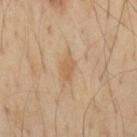Imaged during a routine full-body skin examination; the lesion was not biopsied and no histopathology is available. From the mid back. About 2.5 mm across. The subject is a male in their mid- to late 50s. A roughly 15 mm field-of-view crop from a total-body skin photograph. Automated image analysis of the tile measured a lesion color around L≈59 a*≈18 b*≈36 in CIELAB and a lesion-to-skin contrast of about 6 (normalized; higher = more distinct). And it measured a classifier nevus-likeness of about 10/100 and a detector confidence of about 100 out of 100 that the crop contains a lesion. Captured under cross-polarized illumination.